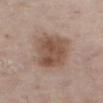subject: male, aged 73 to 77 | body site: the right thigh | image source: 15 mm crop, total-body photography | diameter: ~5 mm (longest diameter) | automated lesion analysis: an outline eccentricity of about 0.55 (0 = round, 1 = elongated) and two-axis asymmetry of about 0.2; border irregularity of about 2 on a 0–10 scale and a peripheral color-asymmetry measure near 1.5; a classifier nevus-likeness of about 60/100 and a lesion-detection confidence of about 100/100.